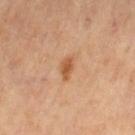Q: Was this lesion biopsied?
A: total-body-photography surveillance lesion; no biopsy
Q: Who is the patient?
A: female, aged around 65
Q: What is the lesion's diameter?
A: about 3 mm
Q: How was the tile lit?
A: cross-polarized
Q: Lesion location?
A: the left thigh
Q: What kind of image is this?
A: 15 mm crop, total-body photography
Q: Automated lesion metrics?
A: a footprint of about 3.5 mm², an eccentricity of roughly 0.85, and two-axis asymmetry of about 0.35; an automated nevus-likeness rating near 80 out of 100 and a lesion-detection confidence of about 100/100